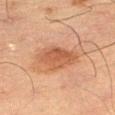Recorded during total-body skin imaging; not selected for excision or biopsy. Approximately 4.5 mm at its widest. The subject is a male in their mid-60s. Cropped from a total-body skin-imaging series; the visible field is about 15 mm. The lesion is located on the right thigh. Automated image analysis of the tile measured a lesion area of about 13 mm², an outline eccentricity of about 0.65 (0 = round, 1 = elongated), and two-axis asymmetry of about 0.2.